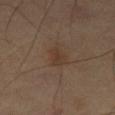Assessment:
No biopsy was performed on this lesion — it was imaged during a full skin examination and was not determined to be concerning.
Image and clinical context:
On the leg. A 15 mm crop from a total-body photograph taken for skin-cancer surveillance. Measured at roughly 3 mm in maximum diameter. An algorithmic analysis of the crop reported an area of roughly 4 mm² and a symmetry-axis asymmetry near 0.25. It also reported border irregularity of about 3 on a 0–10 scale. The analysis additionally found an automated nevus-likeness rating near 15 out of 100 and a detector confidence of about 100 out of 100 that the crop contains a lesion. The tile uses cross-polarized illumination.